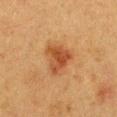notes=no biopsy performed (imaged during a skin exam)
patient=female, aged 38–42
image source=total-body-photography crop, ~15 mm field of view
location=the chest
lesion diameter=~4 mm (longest diameter)
TBP lesion metrics=a lesion color around L≈41 a*≈22 b*≈34 in CIELAB and about 9 CIELAB-L* units darker than the surrounding skin; an automated nevus-likeness rating near 95 out of 100 and a detector confidence of about 100 out of 100 that the crop contains a lesion